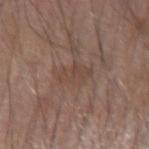Captured during whole-body skin photography for melanoma surveillance; the lesion was not biopsied. Cropped from a whole-body photographic skin survey; the tile spans about 15 mm. A male subject, aged 68–72. Located on the arm. Captured under white-light illumination.